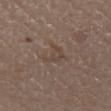Imaged during a routine full-body skin examination; the lesion was not biopsied and no histopathology is available. The patient is a male roughly 80 years of age. A region of skin cropped from a whole-body photographic capture, roughly 15 mm wide. The lesion is on the abdomen.